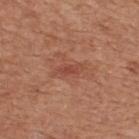{
  "biopsy_status": "not biopsied; imaged during a skin examination",
  "patient": {
    "sex": "male",
    "age_approx": 65
  },
  "site": "upper back",
  "automated_metrics": {
    "area_mm2_approx": 3.5,
    "shape_asymmetry": 0.35,
    "nevus_likeness_0_100": 0
  },
  "lighting": "white-light",
  "image": {
    "source": "total-body photography crop",
    "field_of_view_mm": 15
  }
}